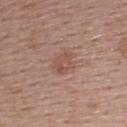biopsy status: imaged on a skin check; not biopsied.